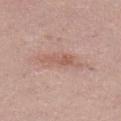{
  "biopsy_status": "not biopsied; imaged during a skin examination",
  "automated_metrics": {
    "area_mm2_approx": 7.0,
    "eccentricity": 0.95,
    "shape_asymmetry": 0.35,
    "vs_skin_darker_L": 8.0,
    "vs_skin_contrast_norm": 6.0,
    "border_irregularity_0_10": 5.5,
    "color_variation_0_10": 2.5,
    "peripheral_color_asymmetry": 1.0
  },
  "image": {
    "source": "total-body photography crop",
    "field_of_view_mm": 15
  },
  "patient": {
    "sex": "female",
    "age_approx": 30
  },
  "lesion_size": {
    "long_diameter_mm_approx": 5.0
  },
  "site": "left lower leg"
}